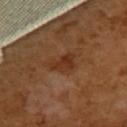{
  "biopsy_status": "not biopsied; imaged during a skin examination",
  "patient": {
    "sex": "female",
    "age_approx": 55
  },
  "site": "upper back",
  "lighting": "cross-polarized",
  "lesion_size": {
    "long_diameter_mm_approx": 3.5
  },
  "image": {
    "source": "total-body photography crop",
    "field_of_view_mm": 15
  }
}